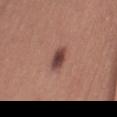Clinical impression:
Recorded during total-body skin imaging; not selected for excision or biopsy.
Image and clinical context:
A female patient, about 30 years old. The recorded lesion diameter is about 3.5 mm. Cropped from a total-body skin-imaging series; the visible field is about 15 mm. The lesion is located on the right thigh. The lesion-visualizer software estimated a lesion area of about 5 mm², an outline eccentricity of about 0.85 (0 = round, 1 = elongated), and a symmetry-axis asymmetry near 0.15. It also reported an average lesion color of about L≈43 a*≈22 b*≈23 (CIELAB), roughly 14 lightness units darker than nearby skin, and a normalized border contrast of about 10.5. The analysis additionally found a nevus-likeness score of about 85/100 and a lesion-detection confidence of about 100/100.